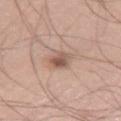follow-up: no biopsy performed (imaged during a skin exam) | lighting: white-light illumination | site: the leg | automated metrics: a lesion area of about 5 mm², a shape eccentricity near 0.7, and a shape-asymmetry score of about 0.2 (0 = symmetric); an automated nevus-likeness rating near 85 out of 100 and a detector confidence of about 100 out of 100 that the crop contains a lesion | patient: male, approximately 40 years of age | lesion diameter: ~3 mm (longest diameter) | image source: ~15 mm crop, total-body skin-cancer survey.